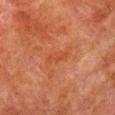biopsy status: no biopsy performed (imaged during a skin exam) | anatomic site: the right lower leg | image source: 15 mm crop, total-body photography | TBP lesion metrics: an area of roughly 3 mm² and a shape-asymmetry score of about 0.25 (0 = symmetric); about 4 CIELAB-L* units darker than the surrounding skin; a nevus-likeness score of about 0/100 and a detector confidence of about 100 out of 100 that the crop contains a lesion | subject: male, approximately 80 years of age | tile lighting: cross-polarized | lesion diameter: ≈3 mm.